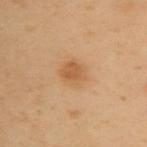workup: imaged on a skin check; not biopsied
automated lesion analysis: a border-irregularity rating of about 2/10, a color-variation rating of about 2/10, and peripheral color asymmetry of about 0.5
patient: female, aged 58 to 62
image source: total-body-photography crop, ~15 mm field of view
site: the upper back
lesion diameter: about 3.5 mm